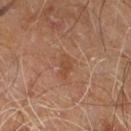| feature | finding |
|---|---|
| workup | no biopsy performed (imaged during a skin exam) |
| patient | male, about 60 years old |
| acquisition | ~15 mm crop, total-body skin-cancer survey |
| automated metrics | a lesion–skin lightness drop of about 6 and a lesion-to-skin contrast of about 6 (normalized; higher = more distinct); border irregularity of about 3 on a 0–10 scale and a color-variation rating of about 1/10 |
| location | the right leg |